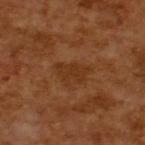| key | value |
|---|---|
| workup | imaged on a skin check; not biopsied |
| lighting | cross-polarized |
| size | ~3.5 mm (longest diameter) |
| imaging modality | ~15 mm crop, total-body skin-cancer survey |
| subject | male, in their mid- to late 60s |